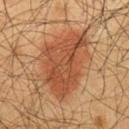| key | value |
|---|---|
| notes | imaged on a skin check; not biopsied |
| acquisition | total-body-photography crop, ~15 mm field of view |
| lesion diameter | about 8 mm |
| body site | the chest |
| subject | male, about 60 years old |
| image-analysis metrics | a mean CIELAB color near L≈45 a*≈24 b*≈34 and a lesion–skin lightness drop of about 10; an automated nevus-likeness rating near 100 out of 100 and a lesion-detection confidence of about 100/100 |